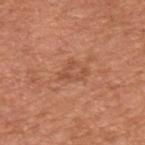| key | value |
|---|---|
| notes | total-body-photography surveillance lesion; no biopsy |
| subject | male, aged around 65 |
| illumination | white-light |
| site | the upper back |
| image source | 15 mm crop, total-body photography |